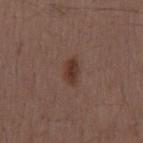Assessment:
Part of a total-body skin-imaging series; this lesion was reviewed on a skin check and was not flagged for biopsy.